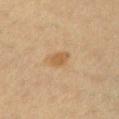Part of a total-body skin-imaging series; this lesion was reviewed on a skin check and was not flagged for biopsy.
Measured at roughly 2.5 mm in maximum diameter.
The patient is a female about 40 years old.
Captured under cross-polarized illumination.
Automated tile analysis of the lesion measured a mean CIELAB color near L≈49 a*≈16 b*≈34, about 7 CIELAB-L* units darker than the surrounding skin, and a normalized lesion–skin contrast near 7.
A close-up tile cropped from a whole-body skin photograph, about 15 mm across.
From the chest.